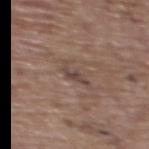Assessment: Part of a total-body skin-imaging series; this lesion was reviewed on a skin check and was not flagged for biopsy. Image and clinical context: The lesion is located on the upper back. A female patient, aged around 65. Cropped from a whole-body photographic skin survey; the tile spans about 15 mm.